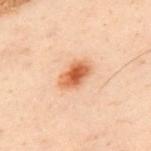<tbp_lesion>
  <biopsy_status>not biopsied; imaged during a skin examination</biopsy_status>
  <image>
    <source>total-body photography crop</source>
    <field_of_view_mm>15</field_of_view_mm>
  </image>
  <automated_metrics>
    <area_mm2_approx>7.0</area_mm2_approx>
    <eccentricity>0.8</eccentricity>
    <shape_asymmetry>0.2</shape_asymmetry>
  </automated_metrics>
  <patient>
    <sex>male</sex>
    <age_approx>50</age_approx>
  </patient>
  <site>upper back</site>
</tbp_lesion>